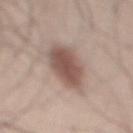Q: Is there a histopathology result?
A: total-body-photography surveillance lesion; no biopsy
Q: Patient demographics?
A: male, roughly 35 years of age
Q: What is the imaging modality?
A: ~15 mm tile from a whole-body skin photo
Q: Where on the body is the lesion?
A: the mid back